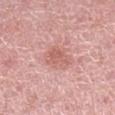Case summary:
* follow-up: total-body-photography surveillance lesion; no biopsy
* image: total-body-photography crop, ~15 mm field of view
* anatomic site: the leg
* patient: female, aged approximately 40
* illumination: white-light illumination
* automated lesion analysis: border irregularity of about 3 on a 0–10 scale and peripheral color asymmetry of about 1
* size: ≈3.5 mm